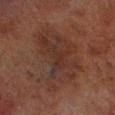| field | value |
|---|---|
| notes | total-body-photography surveillance lesion; no biopsy |